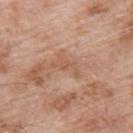| field | value |
|---|---|
| follow-up | imaged on a skin check; not biopsied |
| subject | male, roughly 55 years of age |
| lighting | white-light |
| location | the upper back |
| image | ~15 mm tile from a whole-body skin photo |
| diameter | ≈3.5 mm |
| automated metrics | an outline eccentricity of about 0.9 (0 = round, 1 = elongated); a classifier nevus-likeness of about 0/100 and a detector confidence of about 100 out of 100 that the crop contains a lesion |